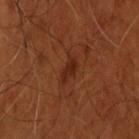Q: Was this lesion biopsied?
A: total-body-photography surveillance lesion; no biopsy
Q: Patient demographics?
A: male, aged around 55
Q: What is the imaging modality?
A: ~15 mm tile from a whole-body skin photo
Q: Where on the body is the lesion?
A: the head or neck